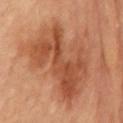Recorded during total-body skin imaging; not selected for excision or biopsy. A region of skin cropped from a whole-body photographic capture, roughly 15 mm wide. A male patient approximately 85 years of age or older. Longest diameter approximately 8.5 mm. The tile uses cross-polarized illumination. An algorithmic analysis of the crop reported a border-irregularity index near 6.5/10, internal color variation of about 5.5 on a 0–10 scale, and radial color variation of about 2. Located on the chest.